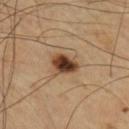{"biopsy_status": "not biopsied; imaged during a skin examination", "image": {"source": "total-body photography crop", "field_of_view_mm": 15}, "site": "upper back", "patient": {"sex": "male", "age_approx": 65}, "lesion_size": {"long_diameter_mm_approx": 3.0}}